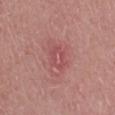biopsy status = total-body-photography surveillance lesion; no biopsy
lighting = white-light
site = the left lower leg
size = ≈3.5 mm
image = total-body-photography crop, ~15 mm field of view
patient = male, aged 68 to 72